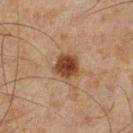biopsy_status: not biopsied; imaged during a skin examination
site: left lower leg
image:
  source: total-body photography crop
  field_of_view_mm: 15
lighting: cross-polarized
lesion_size:
  long_diameter_mm_approx: 3.0
patient:
  sex: male
  age_approx: 45